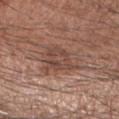The lesion was photographed on a routine skin check and not biopsied; there is no pathology result.
This is a white-light tile.
Automated tile analysis of the lesion measured a footprint of about 11 mm² and two-axis asymmetry of about 0.35. It also reported a classifier nevus-likeness of about 0/100 and a lesion-detection confidence of about 100/100.
Cropped from a whole-body photographic skin survey; the tile spans about 15 mm.
Located on the right forearm.
About 4 mm across.
A male subject, aged approximately 70.